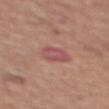patient=male, in their mid-60s; anatomic site=the back; image source=~15 mm crop, total-body skin-cancer survey; tile lighting=white-light illumination.